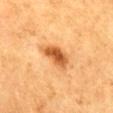Impression: Captured during whole-body skin photography for melanoma surveillance; the lesion was not biopsied. Image and clinical context: The lesion is on the back. A female patient in their mid-50s. The recorded lesion diameter is about 3.5 mm. This is a cross-polarized tile. This image is a 15 mm lesion crop taken from a total-body photograph.